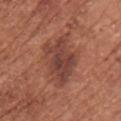Recorded during total-body skin imaging; not selected for excision or biopsy.
This is a white-light tile.
A female subject roughly 75 years of age.
A roughly 15 mm field-of-view crop from a total-body skin photograph.
The total-body-photography lesion software estimated a mean CIELAB color near L≈42 a*≈24 b*≈26, a lesion–skin lightness drop of about 10, and a lesion-to-skin contrast of about 8 (normalized; higher = more distinct). The analysis additionally found an automated nevus-likeness rating near 50 out of 100 and a lesion-detection confidence of about 100/100.
The lesion is on the upper back.
The recorded lesion diameter is about 6.5 mm.